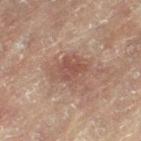Q: Is there a histopathology result?
A: no biopsy performed (imaged during a skin exam)
Q: Patient demographics?
A: female, roughly 80 years of age
Q: What is the imaging modality?
A: 15 mm crop, total-body photography
Q: What is the anatomic site?
A: the right leg
Q: What lighting was used for the tile?
A: cross-polarized